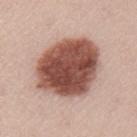Findings:
- size: ≈8 mm
- tile lighting: white-light
- image: total-body-photography crop, ~15 mm field of view
- patient: female, in their 50s
- automated lesion analysis: a shape eccentricity near 0.65 and two-axis asymmetry of about 0.1; an average lesion color of about L≈50 a*≈23 b*≈26 (CIELAB) and a normalized lesion–skin contrast near 13.5; a border-irregularity index near 1.5/10; a classifier nevus-likeness of about 90/100 and a detector confidence of about 100 out of 100 that the crop contains a lesion
- site: the left upper arm